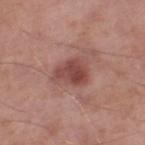Imaged during a routine full-body skin examination; the lesion was not biopsied and no histopathology is available. A region of skin cropped from a whole-body photographic capture, roughly 15 mm wide. This is a white-light tile. Located on the leg. The recorded lesion diameter is about 4 mm. The subject is a male aged 53–57.